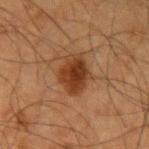Q: Was this lesion biopsied?
A: total-body-photography surveillance lesion; no biopsy
Q: What kind of image is this?
A: 15 mm crop, total-body photography
Q: How large is the lesion?
A: about 4.5 mm
Q: Where on the body is the lesion?
A: the left upper arm
Q: How was the tile lit?
A: cross-polarized
Q: Who is the patient?
A: male, aged 63–67